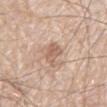No biopsy was performed on this lesion — it was imaged during a full skin examination and was not determined to be concerning.
The lesion-visualizer software estimated a lesion area of about 5.5 mm² and an outline eccentricity of about 0.85 (0 = round, 1 = elongated). It also reported a mean CIELAB color near L≈61 a*≈18 b*≈29, roughly 10 lightness units darker than nearby skin, and a normalized lesion–skin contrast near 6.5.
This is a white-light tile.
On the mid back.
The subject is a male aged approximately 80.
A lesion tile, about 15 mm wide, cut from a 3D total-body photograph.
The lesion's longest dimension is about 3.5 mm.